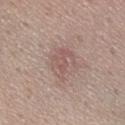biopsy status = catalogued during a skin exam; not biopsied
patient = female, aged 48–52
anatomic site = the chest
imaging modality = ~15 mm crop, total-body skin-cancer survey
lesion size = ~4 mm (longest diameter)